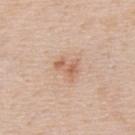Findings:
* notes — imaged on a skin check; not biopsied
* acquisition — total-body-photography crop, ~15 mm field of view
* body site — the upper back
* patient — male, aged 48 to 52